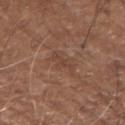follow-up=catalogued during a skin exam; not biopsied
illumination=white-light illumination
anatomic site=the left forearm
image=~15 mm crop, total-body skin-cancer survey
patient=male, aged 73–77
TBP lesion metrics=a border-irregularity index near 6/10 and a within-lesion color-variation index near 0/10; a lesion-detection confidence of about 100/100